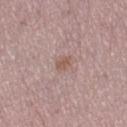From the left thigh. The total-body-photography lesion software estimated a lesion color around L≈56 a*≈19 b*≈24 in CIELAB, roughly 7 lightness units darker than nearby skin, and a lesion-to-skin contrast of about 6 (normalized; higher = more distinct). The software also gave a border-irregularity index near 2.5/10. It also reported an automated nevus-likeness rating near 30 out of 100. The subject is a male roughly 60 years of age. Imaged with white-light lighting. A 15 mm close-up extracted from a 3D total-body photography capture.